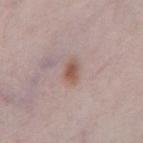notes: catalogued during a skin exam; not biopsied
image source: ~15 mm tile from a whole-body skin photo
tile lighting: white-light illumination
site: the front of the torso
automated lesion analysis: a lesion area of about 3.5 mm², an eccentricity of roughly 0.8, and a symmetry-axis asymmetry near 0.2; about 10 CIELAB-L* units darker than the surrounding skin and a lesion-to-skin contrast of about 8.5 (normalized; higher = more distinct); a border-irregularity index near 2/10 and internal color variation of about 3 on a 0–10 scale
patient: male, aged approximately 70
size: ~2.5 mm (longest diameter)